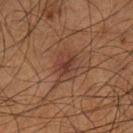This lesion was catalogued during total-body skin photography and was not selected for biopsy.
The lesion is on the left lower leg.
About 4 mm across.
This is a cross-polarized tile.
A male patient about 55 years old.
Cropped from a whole-body photographic skin survey; the tile spans about 15 mm.